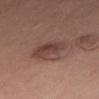workup = imaged on a skin check; not biopsied | illumination = white-light | site = the abdomen | lesion size = about 4.5 mm | imaging modality = ~15 mm tile from a whole-body skin photo | subject = male, aged approximately 40.